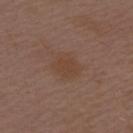Part of a total-body skin-imaging series; this lesion was reviewed on a skin check and was not flagged for biopsy.
On the upper back.
The patient is a male approximately 50 years of age.
An algorithmic analysis of the crop reported internal color variation of about 1.5 on a 0–10 scale and a peripheral color-asymmetry measure near 0.5. And it measured a classifier nevus-likeness of about 0/100 and a lesion-detection confidence of about 100/100.
A roughly 15 mm field-of-view crop from a total-body skin photograph.
The lesion's longest dimension is about 3.5 mm.
The tile uses white-light illumination.